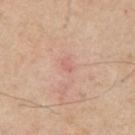{"biopsy_status": "not biopsied; imaged during a skin examination", "site": "right upper arm", "image": {"source": "total-body photography crop", "field_of_view_mm": 15}, "lesion_size": {"long_diameter_mm_approx": 1.5}, "automated_metrics": {"cielab_L": 62, "cielab_a": 26, "cielab_b": 28, "vs_skin_darker_L": 6.0, "vs_skin_contrast_norm": 4.0, "border_irregularity_0_10": 3.5, "color_variation_0_10": 0.0, "nevus_likeness_0_100": 0, "lesion_detection_confidence_0_100": 100}, "patient": {"sex": "male", "age_approx": 55}, "lighting": "white-light"}